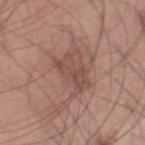Recorded during total-body skin imaging; not selected for excision or biopsy. From the right lower leg. A male subject, aged around 45. This is a white-light tile. Measured at roughly 8.5 mm in maximum diameter. A lesion tile, about 15 mm wide, cut from a 3D total-body photograph.